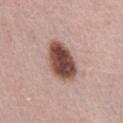<lesion>
<automated_metrics>
  <border_irregularity_0_10>1.5</border_irregularity_0_10>
  <color_variation_0_10>5.5</color_variation_0_10>
  <nevus_likeness_0_100>95</nevus_likeness_0_100>
  <lesion_detection_confidence_0_100>100</lesion_detection_confidence_0_100>
</automated_metrics>
<site>mid back</site>
<image>
  <source>total-body photography crop</source>
  <field_of_view_mm>15</field_of_view_mm>
</image>
<lesion_size>
  <long_diameter_mm_approx>5.0</long_diameter_mm_approx>
</lesion_size>
<lighting>white-light</lighting>
<patient>
  <sex>male</sex>
  <age_approx>65</age_approx>
</patient>
</lesion>